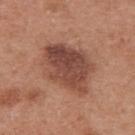Recorded during total-body skin imaging; not selected for excision or biopsy. This is a white-light tile. On the upper back. Cropped from a whole-body photographic skin survey; the tile spans about 15 mm. A female subject in their 40s.